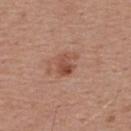The lesion was tiled from a total-body skin photograph and was not biopsied. A 15 mm close-up extracted from a 3D total-body photography capture. A male subject, approximately 55 years of age. Captured under white-light illumination. The lesion's longest dimension is about 2.5 mm. Located on the upper back.